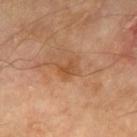This lesion was catalogued during total-body skin photography and was not selected for biopsy.
A 15 mm crop from a total-body photograph taken for skin-cancer surveillance.
The lesion is on the leg.
A male subject, aged 68–72.